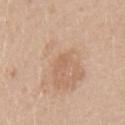<record>
<biopsy_status>not biopsied; imaged during a skin examination</biopsy_status>
<patient>
  <sex>female</sex>
  <age_approx>20</age_approx>
</patient>
<site>left upper arm</site>
<image>
  <source>total-body photography crop</source>
  <field_of_view_mm>15</field_of_view_mm>
</image>
</record>